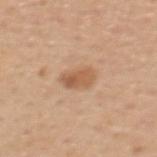This lesion was catalogued during total-body skin photography and was not selected for biopsy.